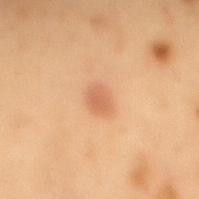Recorded during total-body skin imaging; not selected for excision or biopsy.
Cropped from a total-body skin-imaging series; the visible field is about 15 mm.
The lesion's longest dimension is about 2.5 mm.
A male subject aged approximately 55.
Automated tile analysis of the lesion measured border irregularity of about 2 on a 0–10 scale and radial color variation of about 0.5. The software also gave a nevus-likeness score of about 85/100 and lesion-presence confidence of about 100/100.
The lesion is located on the mid back.